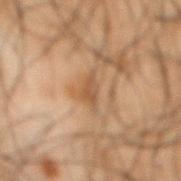biopsy_status: not biopsied; imaged during a skin examination
site: mid back
patient:
  sex: male
  age_approx: 50
image:
  source: total-body photography crop
  field_of_view_mm: 15
automated_metrics:
  border_irregularity_0_10: 3.5
  color_variation_0_10: 1.5
  peripheral_color_asymmetry: 0.5
lighting: cross-polarized
lesion_size:
  long_diameter_mm_approx: 2.5